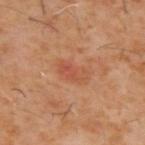| feature | finding |
|---|---|
| notes | total-body-photography surveillance lesion; no biopsy |
| imaging modality | 15 mm crop, total-body photography |
| automated lesion analysis | a footprint of about 6.5 mm², a shape eccentricity near 0.85, and a shape-asymmetry score of about 0.2 (0 = symmetric) |
| size | ~3.5 mm (longest diameter) |
| body site | the upper back |
| subject | male, aged 58 to 62 |
| tile lighting | cross-polarized illumination |